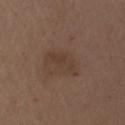Findings:
– follow-up — imaged on a skin check; not biopsied
– patient — male, roughly 70 years of age
– site — the arm
– tile lighting — white-light
– lesion size — about 5 mm
– imaging modality — ~15 mm crop, total-body skin-cancer survey